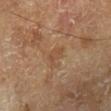image = ~15 mm crop, total-body skin-cancer survey
lesion diameter = ≈2.5 mm
illumination = cross-polarized illumination
site = the leg
subject = male, aged 63 to 67